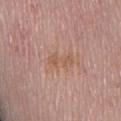Clinical impression: Imaged during a routine full-body skin examination; the lesion was not biopsied and no histopathology is available. Clinical summary: About 3 mm across. This is a white-light tile. A male patient roughly 75 years of age. Cropped from a whole-body photographic skin survey; the tile spans about 15 mm. The lesion is located on the mid back. Automated image analysis of the tile measured an average lesion color of about L≈56 a*≈20 b*≈29 (CIELAB), about 6 CIELAB-L* units darker than the surrounding skin, and a normalized lesion–skin contrast near 5.